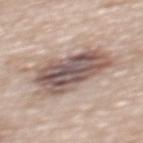follow-up=catalogued during a skin exam; not biopsied | TBP lesion metrics=a lesion color around L≈54 a*≈16 b*≈21 in CIELAB, a lesion–skin lightness drop of about 15, and a normalized lesion–skin contrast near 10.5 | lesion diameter=~7 mm (longest diameter) | anatomic site=the upper back | image source=total-body-photography crop, ~15 mm field of view | subject=male, about 70 years old | tile lighting=white-light.